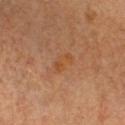Context: A 15 mm close-up extracted from a 3D total-body photography capture. Located on the chest. The lesion-visualizer software estimated a footprint of about 4.5 mm², an outline eccentricity of about 0.8 (0 = round, 1 = elongated), and a shape-asymmetry score of about 0.35 (0 = symmetric). The analysis additionally found roughly 4 lightness units darker than nearby skin and a normalized border contrast of about 5.5. And it measured a border-irregularity rating of about 3.5/10, internal color variation of about 3 on a 0–10 scale, and radial color variation of about 1. Imaged with cross-polarized lighting. A male patient aged around 65.